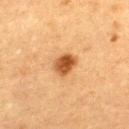Case summary:
– notes: no biopsy performed (imaged during a skin exam)
– patient: female, about 55 years old
– imaging modality: ~15 mm crop, total-body skin-cancer survey
– anatomic site: the upper back